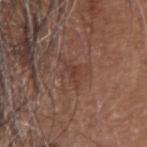This is a white-light tile. From the head or neck. The subject is a male aged 68–72. Automated tile analysis of the lesion measured an area of roughly 2.5 mm² and a symmetry-axis asymmetry near 0.45. The recorded lesion diameter is about 2.5 mm. A 15 mm crop from a total-body photograph taken for skin-cancer surveillance.